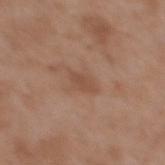  biopsy_status: not biopsied; imaged during a skin examination
  patient:
    sex: female
    age_approx: 35
  site: upper back
  image:
    source: total-body photography crop
    field_of_view_mm: 15
  automated_metrics:
    area_mm2_approx: 3.0
    eccentricity: 0.9
    shape_asymmetry: 0.15
    cielab_L: 48
    cielab_a: 20
    cielab_b: 29
    vs_skin_darker_L: 7.0
    vs_skin_contrast_norm: 5.5
  lighting: white-light